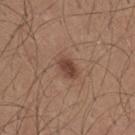Q: Was this lesion biopsied?
A: no biopsy performed (imaged during a skin exam)
Q: What are the patient's age and sex?
A: male, in their mid-20s
Q: How large is the lesion?
A: ~3 mm (longest diameter)
Q: Where on the body is the lesion?
A: the mid back
Q: What is the imaging modality?
A: ~15 mm crop, total-body skin-cancer survey
Q: What lighting was used for the tile?
A: white-light